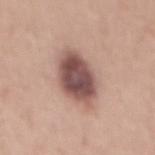biopsy status: total-body-photography surveillance lesion; no biopsy | location: the mid back | acquisition: total-body-photography crop, ~15 mm field of view | subject: male, roughly 30 years of age | lesion diameter: ~6.5 mm (longest diameter) | lighting: white-light.